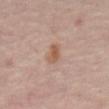Q: Was a biopsy performed?
A: imaged on a skin check; not biopsied
Q: What did automated image analysis measure?
A: a lesion area of about 4 mm², an outline eccentricity of about 0.85 (0 = round, 1 = elongated), and a shape-asymmetry score of about 0.2 (0 = symmetric); an automated nevus-likeness rating near 35 out of 100
Q: How large is the lesion?
A: ~2.5 mm (longest diameter)
Q: What are the patient's age and sex?
A: roughly 55 years of age
Q: How was the tile lit?
A: cross-polarized illumination
Q: What is the anatomic site?
A: the mid back
Q: What kind of image is this?
A: ~15 mm tile from a whole-body skin photo